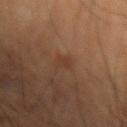workup: total-body-photography surveillance lesion; no biopsy
acquisition: 15 mm crop, total-body photography
patient: male, about 60 years old
body site: the left forearm